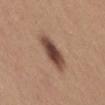| key | value |
|---|---|
| biopsy status | total-body-photography surveillance lesion; no biopsy |
| body site | the right thigh |
| imaging modality | ~15 mm crop, total-body skin-cancer survey |
| patient | female, roughly 25 years of age |
| image-analysis metrics | an area of roughly 10 mm², an eccentricity of roughly 0.85, and a shape-asymmetry score of about 0.2 (0 = symmetric); a mean CIELAB color near L≈46 a*≈20 b*≈26 and a normalized border contrast of about 11; a border-irregularity rating of about 2.5/10, a color-variation rating of about 5/10, and peripheral color asymmetry of about 1.5; a detector confidence of about 100 out of 100 that the crop contains a lesion |
| illumination | white-light illumination |